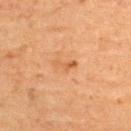<tbp_lesion>
  <biopsy_status>not biopsied; imaged during a skin examination</biopsy_status>
  <lesion_size>
    <long_diameter_mm_approx>3.0</long_diameter_mm_approx>
  </lesion_size>
  <automated_metrics>
    <border_irregularity_0_10>5.0</border_irregularity_0_10>
    <color_variation_0_10>0.0</color_variation_0_10>
    <peripheral_color_asymmetry>0.0</peripheral_color_asymmetry>
  </automated_metrics>
  <image>
    <source>total-body photography crop</source>
    <field_of_view_mm>15</field_of_view_mm>
  </image>
  <site>upper back</site>
  <lighting>cross-polarized</lighting>
  <patient>
    <sex>female</sex>
    <age_approx>70</age_approx>
  </patient>
</tbp_lesion>